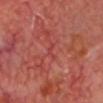The lesion was tiled from a total-body skin photograph and was not biopsied.
Imaged with cross-polarized lighting.
A male patient aged approximately 65.
On the head or neck.
Automated tile analysis of the lesion measured an automated nevus-likeness rating near 0 out of 100.
A region of skin cropped from a whole-body photographic capture, roughly 15 mm wide.
Measured at roughly 3 mm in maximum diameter.